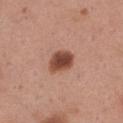  biopsy_status: not biopsied; imaged during a skin examination
  lesion_size:
    long_diameter_mm_approx: 3.5
  site: left thigh
  image:
    source: total-body photography crop
    field_of_view_mm: 15
  patient:
    sex: female
    age_approx: 30